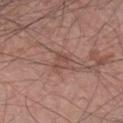{
  "biopsy_status": "not biopsied; imaged during a skin examination",
  "site": "left forearm",
  "image": {
    "source": "total-body photography crop",
    "field_of_view_mm": 15
  },
  "patient": {
    "sex": "male",
    "age_approx": 60
  }
}